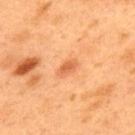Impression: Part of a total-body skin-imaging series; this lesion was reviewed on a skin check and was not flagged for biopsy. Image and clinical context: Longest diameter approximately 2.5 mm. The total-body-photography lesion software estimated a mean CIELAB color near L≈64 a*≈31 b*≈44 and a normalized border contrast of about 6.5. The software also gave a lesion-detection confidence of about 100/100. This is a cross-polarized tile. The lesion is on the upper back. A male patient, aged 48 to 52. A region of skin cropped from a whole-body photographic capture, roughly 15 mm wide.